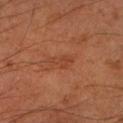biopsy status=total-body-photography surveillance lesion; no biopsy
imaging modality=15 mm crop, total-body photography
lesion diameter=~3.5 mm (longest diameter)
TBP lesion metrics=a lesion area of about 4.5 mm², a shape eccentricity near 0.9, and two-axis asymmetry of about 0.3; a lesion color around L≈39 a*≈25 b*≈31 in CIELAB and a normalized lesion–skin contrast near 5
location=the arm
patient=male, aged 58–62